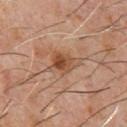| field | value |
|---|---|
| notes | imaged on a skin check; not biopsied |
| subject | male, aged around 60 |
| imaging modality | total-body-photography crop, ~15 mm field of view |
| image-analysis metrics | an eccentricity of roughly 0.7 and a symmetry-axis asymmetry near 0.35; a lesion–skin lightness drop of about 9 and a normalized lesion–skin contrast near 7.5; border irregularity of about 4 on a 0–10 scale, a color-variation rating of about 6.5/10, and radial color variation of about 2 |
| body site | the front of the torso |
| lesion size | about 4 mm |
| lighting | cross-polarized |